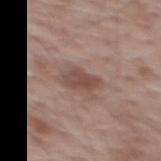lighting=white-light | diameter=≈4 mm | anatomic site=the upper back | automated lesion analysis=a shape eccentricity near 0.85 and two-axis asymmetry of about 0.3; peripheral color asymmetry of about 1; a classifier nevus-likeness of about 5/100 | imaging modality=~15 mm crop, total-body skin-cancer survey | subject=male, about 70 years old.